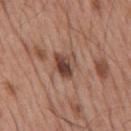The lesion was photographed on a routine skin check and not biopsied; there is no pathology result. Cropped from a whole-body photographic skin survey; the tile spans about 15 mm. An algorithmic analysis of the crop reported a footprint of about 6.5 mm², an eccentricity of roughly 0.65, and a symmetry-axis asymmetry near 0.3. It also reported a border-irregularity index near 3/10. The tile uses white-light illumination. On the mid back. A male subject, roughly 55 years of age.